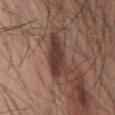– biopsy status: imaged on a skin check; not biopsied
– patient: male, about 55 years old
– TBP lesion metrics: a lesion area of about 11 mm² and an eccentricity of roughly 0.85; border irregularity of about 4.5 on a 0–10 scale, internal color variation of about 3 on a 0–10 scale, and radial color variation of about 1; a classifier nevus-likeness of about 85/100 and lesion-presence confidence of about 100/100
– image: ~15 mm crop, total-body skin-cancer survey
– site: the front of the torso
– size: ~6 mm (longest diameter)
– lighting: white-light